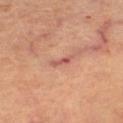Impression:
Recorded during total-body skin imaging; not selected for excision or biopsy.
Acquisition and patient details:
A 15 mm close-up tile from a total-body photography series done for melanoma screening. This is a cross-polarized tile. Measured at roughly 3 mm in maximum diameter. The patient is in their 60s. The lesion is on the left thigh.